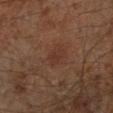The lesion was photographed on a routine skin check and not biopsied; there is no pathology result. A male patient, approximately 60 years of age. Automated image analysis of the tile measured a border-irregularity rating of about 2/10, internal color variation of about 1.5 on a 0–10 scale, and peripheral color asymmetry of about 0.5. The analysis additionally found a lesion-detection confidence of about 100/100. A roughly 15 mm field-of-view crop from a total-body skin photograph. This is a cross-polarized tile. Approximately 3 mm at its widest. From the left lower leg.